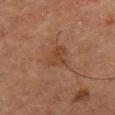notes: no biopsy performed (imaged during a skin exam) | lighting: cross-polarized illumination | acquisition: 15 mm crop, total-body photography | body site: the chest | lesion size: ~3 mm (longest diameter) | patient: male, aged approximately 50.